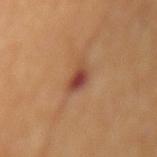Impression: Captured during whole-body skin photography for melanoma surveillance; the lesion was not biopsied. Context: Longest diameter approximately 3 mm. A 15 mm close-up tile from a total-body photography series done for melanoma screening. A male patient, approximately 70 years of age. The lesion is located on the lower back.